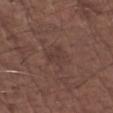Assessment: The lesion was photographed on a routine skin check and not biopsied; there is no pathology result. Acquisition and patient details: Cropped from a whole-body photographic skin survey; the tile spans about 15 mm. The subject is a male in their mid-70s. The lesion is on the left forearm.